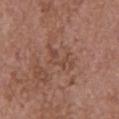workup: no biopsy performed (imaged during a skin exam) | tile lighting: white-light illumination | subject: female, approximately 65 years of age | image source: ~15 mm crop, total-body skin-cancer survey | automated lesion analysis: an area of roughly 4.5 mm² and an outline eccentricity of about 0.9 (0 = round, 1 = elongated); about 6 CIELAB-L* units darker than the surrounding skin and a normalized lesion–skin contrast near 5; an automated nevus-likeness rating near 0 out of 100 | site: the chest | size: about 4 mm.